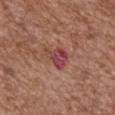  biopsy_status: not biopsied; imaged during a skin examination
  image:
    source: total-body photography crop
    field_of_view_mm: 15
  lighting: white-light
  patient:
    sex: male
    age_approx: 75
  site: chest